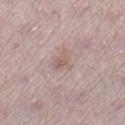Imaged during a routine full-body skin examination; the lesion was not biopsied and no histopathology is available.